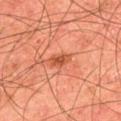This lesion was catalogued during total-body skin photography and was not selected for biopsy.
A lesion tile, about 15 mm wide, cut from a 3D total-body photograph.
The subject is a male aged around 45.
Approximately 2.5 mm at its widest.
From the upper back.
Captured under cross-polarized illumination.